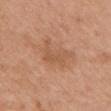biopsy status = catalogued during a skin exam; not biopsied | subject = female, roughly 40 years of age | image source = total-body-photography crop, ~15 mm field of view | lesion diameter = ~3.5 mm (longest diameter) | site = the right upper arm.